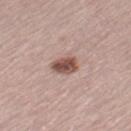follow-up: imaged on a skin check; not biopsied
subject: male, in their mid-60s
site: the left thigh
imaging modality: ~15 mm tile from a whole-body skin photo